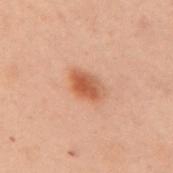  site: upper back
  patient:
    sex: female
    age_approx: 55
  image:
    source: total-body photography crop
    field_of_view_mm: 15